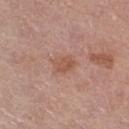<record>
  <biopsy_status>not biopsied; imaged during a skin examination</biopsy_status>
  <lesion_size>
    <long_diameter_mm_approx>3.0</long_diameter_mm_approx>
  </lesion_size>
  <patient>
    <sex>female</sex>
    <age_approx>55</age_approx>
  </patient>
  <image>
    <source>total-body photography crop</source>
    <field_of_view_mm>15</field_of_view_mm>
  </image>
  <site>right thigh</site>
</record>